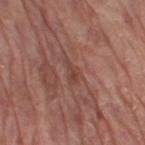  biopsy_status: not biopsied; imaged during a skin examination
  automated_metrics:
    area_mm2_approx: 3.0
    eccentricity: 0.9
    shape_asymmetry: 0.55
  site: leg
  image:
    source: total-body photography crop
    field_of_view_mm: 15
  lesion_size:
    long_diameter_mm_approx: 3.0
  patient:
    sex: male
    age_approx: 70